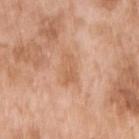diameter: about 3.5 mm | automated lesion analysis: an area of roughly 5 mm² and an eccentricity of roughly 0.85; about 7 CIELAB-L* units darker than the surrounding skin and a normalized border contrast of about 5.5; a border-irregularity index near 4/10 | patient: female, roughly 75 years of age | body site: the right upper arm | acquisition: 15 mm crop, total-body photography | tile lighting: white-light.